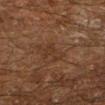Case summary:
* follow-up — catalogued during a skin exam; not biopsied
* lesion size — about 3 mm
* location — the left lower leg
* image source — total-body-photography crop, ~15 mm field of view
* patient — male, approximately 60 years of age
* tile lighting — cross-polarized illumination